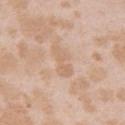<lesion>
  <biopsy_status>not biopsied; imaged during a skin examination</biopsy_status>
  <site>right upper arm</site>
  <patient>
    <sex>female</sex>
    <age_approx>25</age_approx>
  </patient>
  <image>
    <source>total-body photography crop</source>
    <field_of_view_mm>15</field_of_view_mm>
  </image>
  <automated_metrics>
    <cielab_L>65</cielab_L>
    <cielab_a>17</cielab_a>
    <cielab_b>32</cielab_b>
    <vs_skin_darker_L>6.0</vs_skin_darker_L>
    <vs_skin_contrast_norm>5.0</vs_skin_contrast_norm>
    <border_irregularity_0_10>5.5</border_irregularity_0_10>
  </automated_metrics>
</lesion>